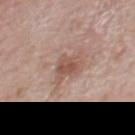Captured during whole-body skin photography for melanoma surveillance; the lesion was not biopsied. A male subject, aged 68 to 72. The total-body-photography lesion software estimated an area of roughly 6 mm², a shape eccentricity near 0.6, and a symmetry-axis asymmetry near 0.35. And it measured a lesion color around L≈53 a*≈21 b*≈26 in CIELAB and a lesion-to-skin contrast of about 6.5 (normalized; higher = more distinct). The software also gave a detector confidence of about 100 out of 100 that the crop contains a lesion. A 15 mm crop from a total-body photograph taken for skin-cancer surveillance. From the chest. Longest diameter approximately 3.5 mm. The tile uses white-light illumination.